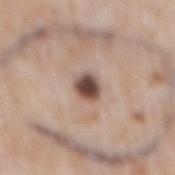A 15 mm crop from a total-body photograph taken for skin-cancer surveillance. Imaged with white-light lighting. A male subject aged approximately 50. On the mid back.